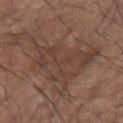Captured during whole-body skin photography for melanoma surveillance; the lesion was not biopsied. The lesion's longest dimension is about 11 mm. A male patient, approximately 80 years of age. Automated image analysis of the tile measured a footprint of about 28 mm² and two-axis asymmetry of about 0.5. And it measured a lesion color around L≈40 a*≈17 b*≈24 in CIELAB, about 7 CIELAB-L* units darker than the surrounding skin, and a lesion-to-skin contrast of about 6 (normalized; higher = more distinct). It also reported a classifier nevus-likeness of about 0/100. Cropped from a total-body skin-imaging series; the visible field is about 15 mm. On the right upper arm.